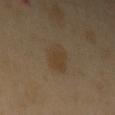follow-up = no biopsy performed (imaged during a skin exam); acquisition = ~15 mm crop, total-body skin-cancer survey; patient = male, aged 38 to 42; size = ≈4 mm; anatomic site = the left upper arm.